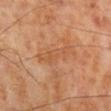Impression: Part of a total-body skin-imaging series; this lesion was reviewed on a skin check and was not flagged for biopsy. Acquisition and patient details: Located on the right lower leg. Automated image analysis of the tile measured a mean CIELAB color near L≈53 a*≈23 b*≈37, about 6 CIELAB-L* units darker than the surrounding skin, and a normalized lesion–skin contrast near 4.5. This is a cross-polarized tile. A 15 mm crop from a total-body photograph taken for skin-cancer surveillance. A male patient about 70 years old. The lesion's longest dimension is about 5.5 mm.